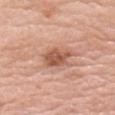Q: Was a biopsy performed?
A: imaged on a skin check; not biopsied
Q: How was this image acquired?
A: ~15 mm crop, total-body skin-cancer survey
Q: Lesion location?
A: the right upper arm
Q: Who is the patient?
A: male, approximately 80 years of age
Q: What lighting was used for the tile?
A: white-light illumination